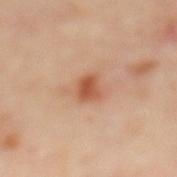Q: Was a biopsy performed?
A: catalogued during a skin exam; not biopsied
Q: Illumination type?
A: cross-polarized illumination
Q: Where on the body is the lesion?
A: the back
Q: How large is the lesion?
A: about 2.5 mm
Q: What kind of image is this?
A: total-body-photography crop, ~15 mm field of view
Q: Patient demographics?
A: female, about 60 years old